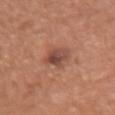Assessment: This lesion was catalogued during total-body skin photography and was not selected for biopsy.